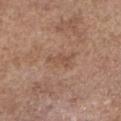biopsy status: no biopsy performed (imaged during a skin exam)
imaging modality: ~15 mm tile from a whole-body skin photo
image-analysis metrics: a footprint of about 3 mm² and an eccentricity of roughly 0.9; roughly 6 lightness units darker than nearby skin and a normalized border contrast of about 5; a border-irregularity index near 5.5/10 and a color-variation rating of about 0.5/10
subject: female, in their mid-60s
illumination: white-light
anatomic site: the arm